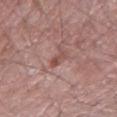Impression:
Recorded during total-body skin imaging; not selected for excision or biopsy.
Acquisition and patient details:
A male subject, in their mid- to late 60s. The tile uses white-light illumination. Longest diameter approximately 2.5 mm. On the right forearm. This image is a 15 mm lesion crop taken from a total-body photograph.